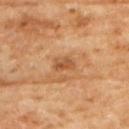biopsy status: catalogued during a skin exam; not biopsied | imaging modality: 15 mm crop, total-body photography | subject: female, approximately 60 years of age | body site: the upper back.